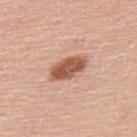Assessment: The lesion was tiled from a total-body skin photograph and was not biopsied. Acquisition and patient details: A close-up tile cropped from a whole-body skin photograph, about 15 mm across. Measured at roughly 4.5 mm in maximum diameter. The lesion is located on the back. The lesion-visualizer software estimated a lesion area of about 9 mm², a shape eccentricity near 0.85, and a symmetry-axis asymmetry near 0.15. The software also gave a border-irregularity rating of about 2/10. And it measured an automated nevus-likeness rating near 95 out of 100 and a detector confidence of about 100 out of 100 that the crop contains a lesion. A male subject aged approximately 60. Imaged with white-light lighting.